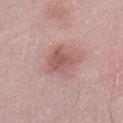Assessment: The lesion was tiled from a total-body skin photograph and was not biopsied. Context: A male subject aged 53 to 57. A lesion tile, about 15 mm wide, cut from a 3D total-body photograph. The lesion is located on the lower back.